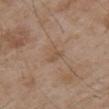This lesion was catalogued during total-body skin photography and was not selected for biopsy. A close-up tile cropped from a whole-body skin photograph, about 15 mm across. The lesion is on the right upper arm. The recorded lesion diameter is about 3 mm. Captured under white-light illumination. A male patient, aged 53 to 57.